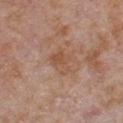Case summary:
- follow-up: imaged on a skin check; not biopsied
- acquisition: 15 mm crop, total-body photography
- diameter: about 3 mm
- illumination: white-light illumination
- patient: male, roughly 65 years of age
- body site: the chest